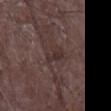| feature | finding |
|---|---|
| notes | catalogued during a skin exam; not biopsied |
| diameter | about 3.5 mm |
| anatomic site | the left forearm |
| subject | male, aged approximately 65 |
| image source | 15 mm crop, total-body photography |
| image-analysis metrics | a normalized lesion–skin contrast near 7 |